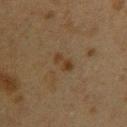Imaged during a routine full-body skin examination; the lesion was not biopsied and no histopathology is available. A roughly 15 mm field-of-view crop from a total-body skin photograph. The subject is a female approximately 40 years of age. This is a cross-polarized tile. The lesion is located on the arm. Longest diameter approximately 3 mm.